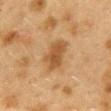Recorded during total-body skin imaging; not selected for excision or biopsy.
About 4.5 mm across.
Cropped from a whole-body photographic skin survey; the tile spans about 15 mm.
Automated tile analysis of the lesion measured a lesion area of about 9.5 mm², an eccentricity of roughly 0.75, and a symmetry-axis asymmetry near 0.2. The analysis additionally found an average lesion color of about L≈44 a*≈17 b*≈34 (CIELAB), a lesion–skin lightness drop of about 9, and a lesion-to-skin contrast of about 7.5 (normalized; higher = more distinct). It also reported a border-irregularity index near 2.5/10, a within-lesion color-variation index near 2.5/10, and a peripheral color-asymmetry measure near 1. And it measured a nevus-likeness score of about 80/100 and lesion-presence confidence of about 100/100.
This is a cross-polarized tile.
A female patient aged around 40.
The lesion is located on the mid back.